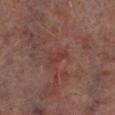workup: imaged on a skin check; not biopsied | patient: male, aged around 65 | lesion diameter: ~3 mm (longest diameter) | anatomic site: the left lower leg | acquisition: ~15 mm crop, total-body skin-cancer survey | image-analysis metrics: a mean CIELAB color near L≈36 a*≈24 b*≈23, roughly 5 lightness units darker than nearby skin, and a normalized border contrast of about 5; a nevus-likeness score of about 0/100.